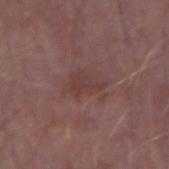lighting: white-light
imaging modality: ~15 mm tile from a whole-body skin photo
automated lesion analysis: a border-irregularity index near 6.5/10 and a color-variation rating of about 1/10
body site: the arm
size: ~3 mm (longest diameter)
patient: male, about 50 years old